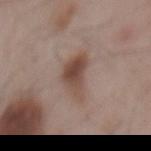The lesion was tiled from a total-body skin photograph and was not biopsied.
The recorded lesion diameter is about 5.5 mm.
A 15 mm crop from a total-body photograph taken for skin-cancer surveillance.
A male subject aged 53 to 57.
The lesion is on the mid back.
The total-body-photography lesion software estimated border irregularity of about 4.5 on a 0–10 scale, internal color variation of about 6 on a 0–10 scale, and peripheral color asymmetry of about 2.5. The analysis additionally found a nevus-likeness score of about 50/100.